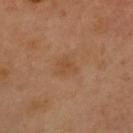| feature | finding |
|---|---|
| notes | catalogued during a skin exam; not biopsied |
| automated lesion analysis | a footprint of about 4.5 mm², a shape eccentricity near 0.45, and two-axis asymmetry of about 0.25; an average lesion color of about L≈41 a*≈18 b*≈30 (CIELAB) and a lesion–skin lightness drop of about 5; a border-irregularity index near 2/10, internal color variation of about 1.5 on a 0–10 scale, and radial color variation of about 0.5; lesion-presence confidence of about 100/100 |
| patient | female, aged 58 to 62 |
| illumination | cross-polarized |
| location | the chest |
| acquisition | 15 mm crop, total-body photography |